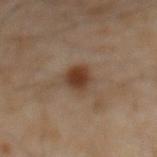Image and clinical context: A close-up tile cropped from a whole-body skin photograph, about 15 mm across. A male patient, in their 60s. From the abdomen.